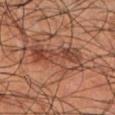{
  "biopsy_status": "not biopsied; imaged during a skin examination",
  "image": {
    "source": "total-body photography crop",
    "field_of_view_mm": 15
  },
  "lighting": "cross-polarized",
  "site": "left lower leg",
  "patient": {
    "sex": "male",
    "age_approx": 55
  }
}